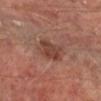Assessment: No biopsy was performed on this lesion — it was imaged during a full skin examination and was not determined to be concerning. Acquisition and patient details: Measured at roughly 3 mm in maximum diameter. Imaged with cross-polarized lighting. The total-body-photography lesion software estimated an average lesion color of about L≈41 a*≈22 b*≈26 (CIELAB), a lesion–skin lightness drop of about 9, and a normalized lesion–skin contrast near 7.5. And it measured a within-lesion color-variation index near 3.5/10. And it measured an automated nevus-likeness rating near 45 out of 100. A male patient in their mid- to late 60s. From the leg. A region of skin cropped from a whole-body photographic capture, roughly 15 mm wide.